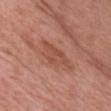Clinical impression: Imaged during a routine full-body skin examination; the lesion was not biopsied and no histopathology is available. Acquisition and patient details: The lesion's longest dimension is about 5.5 mm. Automated image analysis of the tile measured a shape eccentricity near 0.9 and a shape-asymmetry score of about 0.4 (0 = symmetric). The software also gave an average lesion color of about L≈50 a*≈25 b*≈30 (CIELAB), roughly 8 lightness units darker than nearby skin, and a normalized lesion–skin contrast near 5.5. And it measured a border-irregularity index near 5.5/10, a within-lesion color-variation index near 2.5/10, and peripheral color asymmetry of about 1. The lesion is located on the chest. A female patient in their 60s. Cropped from a whole-body photographic skin survey; the tile spans about 15 mm.